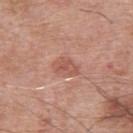{"automated_metrics": {"cielab_L": 55, "cielab_a": 25, "cielab_b": 28, "vs_skin_contrast_norm": 5.5, "color_variation_0_10": 2.5, "peripheral_color_asymmetry": 1.0}, "site": "upper back", "lesion_size": {"long_diameter_mm_approx": 3.0}, "patient": {"sex": "male", "age_approx": 65}, "lighting": "white-light", "image": {"source": "total-body photography crop", "field_of_view_mm": 15}}